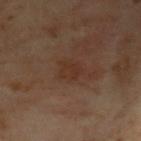Notes:
- workup: no biopsy performed (imaged during a skin exam)
- anatomic site: the upper back
- patient: female, about 60 years old
- image source: ~15 mm crop, total-body skin-cancer survey
- illumination: cross-polarized illumination